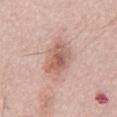location: the chest
illumination: white-light
patient: male, in their mid- to late 50s
automated lesion analysis: roughly 11 lightness units darker than nearby skin; a classifier nevus-likeness of about 15/100 and lesion-presence confidence of about 100/100
diameter: ≈6 mm
image: ~15 mm tile from a whole-body skin photo